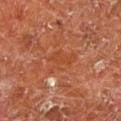Notes:
* follow-up: imaged on a skin check; not biopsied
* lesion size: about 4 mm
* image: ~15 mm tile from a whole-body skin photo
* automated metrics: an automated nevus-likeness rating near 0 out of 100 and lesion-presence confidence of about 60/100
* site: the left lower leg
* lighting: cross-polarized illumination
* patient: male, aged 63–67